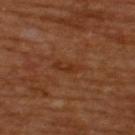Imaged during a routine full-body skin examination; the lesion was not biopsied and no histopathology is available. The tile uses cross-polarized illumination. The recorded lesion diameter is about 3.5 mm. The lesion-visualizer software estimated a lesion area of about 4 mm², an outline eccentricity of about 0.9 (0 = round, 1 = elongated), and a shape-asymmetry score of about 0.3 (0 = symmetric). The analysis additionally found a border-irregularity index near 3.5/10, a color-variation rating of about 1.5/10, and peripheral color asymmetry of about 0.5. It also reported a nevus-likeness score of about 0/100 and a lesion-detection confidence of about 100/100. The lesion is on the upper back. A male patient, in their mid- to late 60s. This image is a 15 mm lesion crop taken from a total-body photograph.